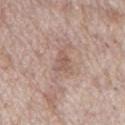Assessment: Imaged during a routine full-body skin examination; the lesion was not biopsied and no histopathology is available. Context: A male subject, roughly 70 years of age. The lesion's longest dimension is about 3 mm. This is a white-light tile. A lesion tile, about 15 mm wide, cut from a 3D total-body photograph. The lesion is on the mid back.